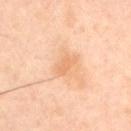{"biopsy_status": "not biopsied; imaged during a skin examination", "image": {"source": "total-body photography crop", "field_of_view_mm": 15}, "lesion_size": {"long_diameter_mm_approx": 3.0}, "site": "chest", "patient": {"sex": "male", "age_approx": 45}, "lighting": "cross-polarized"}